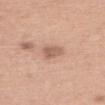Part of a total-body skin-imaging series; this lesion was reviewed on a skin check and was not flagged for biopsy. About 3 mm across. Located on the back. The tile uses white-light illumination. A lesion tile, about 15 mm wide, cut from a 3D total-body photograph. Automated image analysis of the tile measured a footprint of about 4 mm², a shape eccentricity near 0.75, and a symmetry-axis asymmetry near 0.35. It also reported border irregularity of about 3.5 on a 0–10 scale and radial color variation of about 1. The patient is a female about 60 years old.